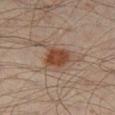The lesion's longest dimension is about 3.5 mm.
A male subject, about 45 years old.
The tile uses cross-polarized illumination.
A 15 mm close-up tile from a total-body photography series done for melanoma screening.
The lesion is located on the right lower leg.